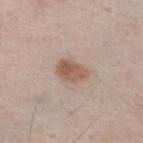image:
  source: total-body photography crop
  field_of_view_mm: 15
patient:
  sex: male
  age_approx: 60
site: right thigh
automated_metrics:
  nevus_likeness_0_100: 90
  lesion_detection_confidence_0_100: 100
lesion_size:
  long_diameter_mm_approx: 3.5
lighting: white-light